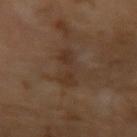No biopsy was performed on this lesion — it was imaged during a full skin examination and was not determined to be concerning. The tile uses cross-polarized illumination. This image is a 15 mm lesion crop taken from a total-body photograph. About 5 mm across. A male subject about 65 years old.